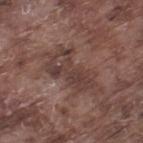The lesion was tiled from a total-body skin photograph and was not biopsied.
Longest diameter approximately 7 mm.
A male subject aged approximately 75.
The tile uses white-light illumination.
Automated image analysis of the tile measured a lesion area of about 16 mm², an outline eccentricity of about 0.9 (0 = round, 1 = elongated), and two-axis asymmetry of about 0.35. The software also gave a mean CIELAB color near L≈39 a*≈17 b*≈21 and a lesion-to-skin contrast of about 6.5 (normalized; higher = more distinct). The analysis additionally found border irregularity of about 5.5 on a 0–10 scale and internal color variation of about 4.5 on a 0–10 scale. And it measured an automated nevus-likeness rating near 0 out of 100 and lesion-presence confidence of about 75/100.
A close-up tile cropped from a whole-body skin photograph, about 15 mm across.
Located on the leg.